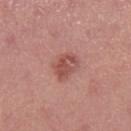  biopsy_status: not biopsied; imaged during a skin examination